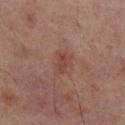Notes:
* notes · imaged on a skin check; not biopsied
* lighting · cross-polarized illumination
* image · ~15 mm tile from a whole-body skin photo
* anatomic site · the mid back
* automated lesion analysis · an area of roughly 4.5 mm², an eccentricity of roughly 0.75, and two-axis asymmetry of about 0.4; roughly 5 lightness units darker than nearby skin and a lesion-to-skin contrast of about 5.5 (normalized; higher = more distinct)
* subject · male, aged around 65